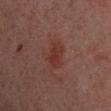This lesion was catalogued during total-body skin photography and was not selected for biopsy. Measured at roughly 3 mm in maximum diameter. The tile uses cross-polarized illumination. A 15 mm close-up tile from a total-body photography series done for melanoma screening. From the left upper arm. Automated tile analysis of the lesion measured an average lesion color of about L≈26 a*≈20 b*≈21 (CIELAB), about 5 CIELAB-L* units darker than the surrounding skin, and a normalized lesion–skin contrast near 7. The software also gave a border-irregularity rating of about 2/10, a within-lesion color-variation index near 3/10, and peripheral color asymmetry of about 1. The software also gave an automated nevus-likeness rating near 40 out of 100 and lesion-presence confidence of about 100/100. A male patient, aged 28–32.